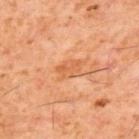Assessment: The lesion was tiled from a total-body skin photograph and was not biopsied. Image and clinical context: Longest diameter approximately 3 mm. An algorithmic analysis of the crop reported an area of roughly 5 mm², an eccentricity of roughly 0.75, and a shape-asymmetry score of about 0.35 (0 = symmetric). The analysis additionally found a lesion color around L≈57 a*≈27 b*≈40 in CIELAB, about 7 CIELAB-L* units darker than the surrounding skin, and a lesion-to-skin contrast of about 5.5 (normalized; higher = more distinct). The analysis additionally found border irregularity of about 3 on a 0–10 scale, a color-variation rating of about 2/10, and a peripheral color-asymmetry measure near 0.5. A male patient, approximately 60 years of age. On the upper back. Cropped from a whole-body photographic skin survey; the tile spans about 15 mm.